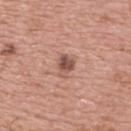Assessment: Recorded during total-body skin imaging; not selected for excision or biopsy. Image and clinical context: This is a white-light tile. On the upper back. A male patient, aged 73 to 77. A 15 mm close-up tile from a total-body photography series done for melanoma screening.